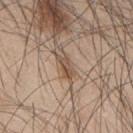notes: total-body-photography surveillance lesion; no biopsy
anatomic site: the upper back
tile lighting: white-light
image: ~15 mm crop, total-body skin-cancer survey
subject: male, aged around 45